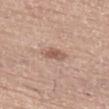This lesion was catalogued during total-body skin photography and was not selected for biopsy.
A 15 mm close-up extracted from a 3D total-body photography capture.
The lesion is on the left thigh.
Imaged with white-light lighting.
The lesion's longest dimension is about 3 mm.
A male subject, roughly 75 years of age.